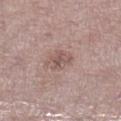Recorded during total-body skin imaging; not selected for excision or biopsy. A roughly 15 mm field-of-view crop from a total-body skin photograph. Approximately 2.5 mm at its widest. The tile uses white-light illumination. The lesion is on the left lower leg. A female patient, about 65 years old.